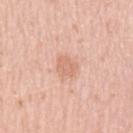Findings:
• follow-up · catalogued during a skin exam; not biopsied
• automated lesion analysis · an area of roughly 4 mm² and two-axis asymmetry of about 0.25; a lesion color around L≈67 a*≈23 b*≈31 in CIELAB, about 8 CIELAB-L* units darker than the surrounding skin, and a normalized border contrast of about 5.5; a border-irregularity index near 2.5/10, internal color variation of about 1 on a 0–10 scale, and radial color variation of about 0.5; a detector confidence of about 100 out of 100 that the crop contains a lesion
• location · the left upper arm
• size · ≈2.5 mm
• acquisition · ~15 mm tile from a whole-body skin photo
• tile lighting · white-light illumination
• patient · female, aged around 30